A roughly 15 mm field-of-view crop from a total-body skin photograph, measured at roughly 3.5 mm in maximum diameter, a female patient in their mid-50s, this is a cross-polarized tile, the lesion is located on the abdomen:
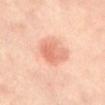pathology: an intradermal melanocytic nevus